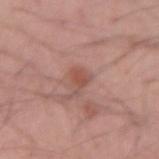Assessment:
The lesion was photographed on a routine skin check and not biopsied; there is no pathology result.
Acquisition and patient details:
The tile uses white-light illumination. The patient is a male in their mid- to late 30s. From the left forearm. The lesion's longest dimension is about 2.5 mm. A 15 mm crop from a total-body photograph taken for skin-cancer surveillance.